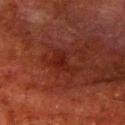Impression:
The lesion was tiled from a total-body skin photograph and was not biopsied.
Background:
The lesion is on the arm. A 15 mm close-up tile from a total-body photography series done for melanoma screening. A male subject in their 80s.